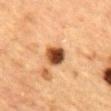| field | value |
|---|---|
| biopsy status | imaged on a skin check; not biopsied |
| body site | the mid back |
| lighting | cross-polarized |
| image source | 15 mm crop, total-body photography |
| subject | male, aged 83 or over |